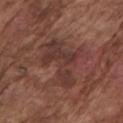Clinical impression:
Imaged during a routine full-body skin examination; the lesion was not biopsied and no histopathology is available.
Image and clinical context:
This image is a 15 mm lesion crop taken from a total-body photograph. The tile uses white-light illumination. The patient is a male aged approximately 75. About 6.5 mm across. The lesion is on the arm.